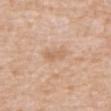Recorded during total-body skin imaging; not selected for excision or biopsy.